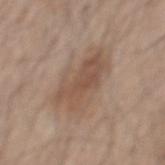The subject is a male about 60 years old. This is a white-light tile. A close-up tile cropped from a whole-body skin photograph, about 15 mm across. The lesion's longest dimension is about 7.5 mm. The lesion is on the mid back.